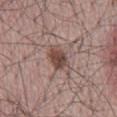<tbp_lesion>
  <biopsy_status>not biopsied; imaged during a skin examination</biopsy_status>
  <image>
    <source>total-body photography crop</source>
    <field_of_view_mm>15</field_of_view_mm>
  </image>
  <site>mid back</site>
  <patient>
    <sex>male</sex>
    <age_approx>65</age_approx>
  </patient>
</tbp_lesion>